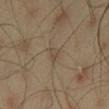{
  "biopsy_status": "not biopsied; imaged during a skin examination",
  "lighting": "cross-polarized",
  "patient": {
    "sex": "male",
    "age_approx": 45
  },
  "image": {
    "source": "total-body photography crop",
    "field_of_view_mm": 15
  },
  "lesion_size": {
    "long_diameter_mm_approx": 2.5
  },
  "site": "left thigh",
  "automated_metrics": {
    "area_mm2_approx": 3.0,
    "eccentricity": 0.85,
    "shape_asymmetry": 0.25,
    "cielab_L": 45,
    "cielab_a": 11,
    "cielab_b": 26,
    "vs_skin_darker_L": 6.0,
    "color_variation_0_10": 2.0,
    "peripheral_color_asymmetry": 1.0
  }
}